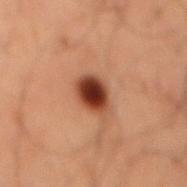This lesion was catalogued during total-body skin photography and was not selected for biopsy. Measured at roughly 3.5 mm in maximum diameter. Cropped from a whole-body photographic skin survey; the tile spans about 15 mm. Automated tile analysis of the lesion measured a footprint of about 7.5 mm², an eccentricity of roughly 0.6, and a symmetry-axis asymmetry near 0.2. The software also gave border irregularity of about 2 on a 0–10 scale, internal color variation of about 5.5 on a 0–10 scale, and a peripheral color-asymmetry measure near 1. The lesion is located on the mid back. A male patient aged approximately 60.